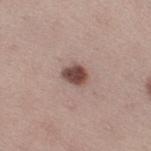Imaged during a routine full-body skin examination; the lesion was not biopsied and no histopathology is available.
On the right thigh.
A female subject, about 45 years old.
A lesion tile, about 15 mm wide, cut from a 3D total-body photograph.
Approximately 3 mm at its widest.
Captured under white-light illumination.